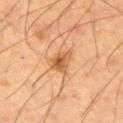The lesion was tiled from a total-body skin photograph and was not biopsied. Automated tile analysis of the lesion measured a lesion color around L≈50 a*≈21 b*≈36 in CIELAB, roughly 11 lightness units darker than nearby skin, and a normalized border contrast of about 8. And it measured border irregularity of about 3.5 on a 0–10 scale, a color-variation rating of about 5/10, and a peripheral color-asymmetry measure near 2. And it measured an automated nevus-likeness rating near 40 out of 100 and a detector confidence of about 100 out of 100 that the crop contains a lesion. The lesion is located on the right upper arm. The patient is a male approximately 55 years of age. A region of skin cropped from a whole-body photographic capture, roughly 15 mm wide.